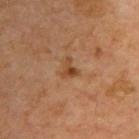Impression:
Recorded during total-body skin imaging; not selected for excision or biopsy.
Context:
A 15 mm crop from a total-body photograph taken for skin-cancer surveillance. The subject is in their mid-60s. The lesion is on the right upper arm. An algorithmic analysis of the crop reported a border-irregularity index near 5.5/10 and radial color variation of about 1.5. The software also gave a classifier nevus-likeness of about 40/100 and lesion-presence confidence of about 100/100. Imaged with cross-polarized lighting. Approximately 2.5 mm at its widest.